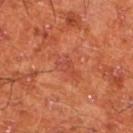{"image": {"source": "total-body photography crop", "field_of_view_mm": 15}, "lesion_size": {"long_diameter_mm_approx": 4.0}, "patient": {"sex": "male", "age_approx": 65}, "automated_metrics": {"eccentricity": 0.85, "shape_asymmetry": 0.35, "nevus_likeness_0_100": 0, "lesion_detection_confidence_0_100": 100}, "lighting": "cross-polarized", "site": "left lower leg"}